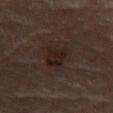Captured during whole-body skin photography for melanoma surveillance; the lesion was not biopsied. An algorithmic analysis of the crop reported a lesion area of about 6.5 mm², a shape eccentricity near 0.7, and a shape-asymmetry score of about 0.25 (0 = symmetric). And it measured a lesion color around L≈19 a*≈13 b*≈17 in CIELAB, roughly 6 lightness units darker than nearby skin, and a lesion-to-skin contrast of about 8 (normalized; higher = more distinct). And it measured border irregularity of about 2.5 on a 0–10 scale, a within-lesion color-variation index near 2.5/10, and radial color variation of about 1. A 15 mm close-up tile from a total-body photography series done for melanoma screening. Imaged with cross-polarized lighting. A male subject, approximately 85 years of age or older. The lesion is located on the left thigh.